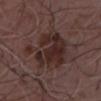Imaged during a routine full-body skin examination; the lesion was not biopsied and no histopathology is available. Automated image analysis of the tile measured a lesion area of about 23 mm² and an eccentricity of roughly 0.15. The software also gave a mean CIELAB color near L≈27 a*≈17 b*≈18. It also reported a border-irregularity rating of about 4.5/10 and a within-lesion color-variation index near 5/10. The lesion is located on the chest. A male subject, in their mid-50s. A lesion tile, about 15 mm wide, cut from a 3D total-body photograph. Imaged with white-light lighting. Approximately 5.5 mm at its widest.